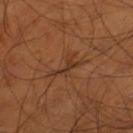A lesion tile, about 15 mm wide, cut from a 3D total-body photograph. The lesion is located on the left thigh. About 4 mm across. Captured under cross-polarized illumination. A male subject in their mid- to late 50s.